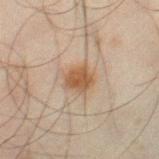* biopsy status · catalogued during a skin exam; not biopsied
* body site · the leg
* tile lighting · cross-polarized illumination
* imaging modality · ~15 mm tile from a whole-body skin photo
* TBP lesion metrics · an average lesion color of about L≈44 a*≈15 b*≈28 (CIELAB), about 9 CIELAB-L* units darker than the surrounding skin, and a normalized lesion–skin contrast near 8.5
* subject · male, aged 43–47
* diameter · ≈3 mm